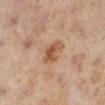  biopsy_status: not biopsied; imaged during a skin examination